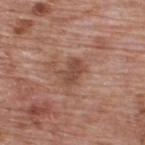Assessment:
The lesion was photographed on a routine skin check and not biopsied; there is no pathology result.
Clinical summary:
The recorded lesion diameter is about 3 mm. A region of skin cropped from a whole-body photographic capture, roughly 15 mm wide. An algorithmic analysis of the crop reported a lesion area of about 5 mm². And it measured a lesion color around L≈47 a*≈22 b*≈27 in CIELAB, a lesion–skin lightness drop of about 9, and a normalized border contrast of about 7. It also reported a border-irregularity rating of about 2.5/10, internal color variation of about 2 on a 0–10 scale, and a peripheral color-asymmetry measure near 0.5. A male subject approximately 70 years of age. On the upper back.